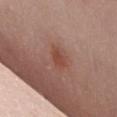Imaged during a routine full-body skin examination; the lesion was not biopsied and no histopathology is available.
A lesion tile, about 15 mm wide, cut from a 3D total-body photograph.
A female subject about 50 years old.
The tile uses white-light illumination.
Located on the chest.